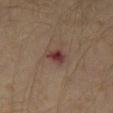Imaged during a routine full-body skin examination; the lesion was not biopsied and no histopathology is available. Imaged with cross-polarized lighting. Cropped from a whole-body photographic skin survey; the tile spans about 15 mm. On the left thigh. A male patient, approximately 30 years of age. Automated image analysis of the tile measured an automated nevus-likeness rating near 0 out of 100. Measured at roughly 3.5 mm in maximum diameter.